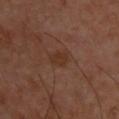notes: imaged on a skin check; not biopsied | tile lighting: cross-polarized illumination | automated metrics: an average lesion color of about L≈31 a*≈19 b*≈27 (CIELAB), a lesion–skin lightness drop of about 5, and a normalized lesion–skin contrast near 6.5; a border-irregularity rating of about 2.5/10, a within-lesion color-variation index near 2/10, and peripheral color asymmetry of about 1 | body site: the back | lesion size: about 2.5 mm | image: ~15 mm crop, total-body skin-cancer survey | patient: male, aged 48 to 52.